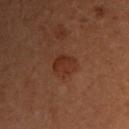location = the upper back
image = total-body-photography crop, ~15 mm field of view
size = ≈3 mm
tile lighting = cross-polarized illumination
patient = female, aged 28 to 32